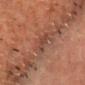Clinical impression: Captured during whole-body skin photography for melanoma surveillance; the lesion was not biopsied. Context: A lesion tile, about 15 mm wide, cut from a 3D total-body photograph. A male subject, about 80 years old. Located on the chest.